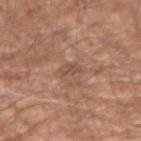Case summary:
* notes: no biopsy performed (imaged during a skin exam)
* subject: male, aged approximately 65
* image: ~15 mm tile from a whole-body skin photo
* body site: the left upper arm